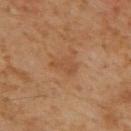Clinical impression: The lesion was photographed on a routine skin check and not biopsied; there is no pathology result. Acquisition and patient details: Imaged with cross-polarized lighting. The subject is a male aged around 60. A 15 mm close-up tile from a total-body photography series done for melanoma screening. The lesion is located on the mid back. Automated tile analysis of the lesion measured a lesion area of about 4.5 mm². It also reported a color-variation rating of about 1.5/10 and radial color variation of about 0.5. Approximately 3 mm at its widest.